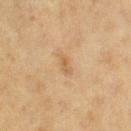Part of a total-body skin-imaging series; this lesion was reviewed on a skin check and was not flagged for biopsy. Longest diameter approximately 2.5 mm. A female patient, aged approximately 50. A 15 mm close-up extracted from a 3D total-body photography capture. The tile uses cross-polarized illumination. From the left thigh.